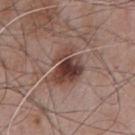Imaged during a routine full-body skin examination; the lesion was not biopsied and no histopathology is available.
On the chest.
Automated tile analysis of the lesion measured a mean CIELAB color near L≈41 a*≈20 b*≈23 and roughly 14 lightness units darker than nearby skin. It also reported an automated nevus-likeness rating near 95 out of 100 and lesion-presence confidence of about 100/100.
A male subject, aged 63 to 67.
Imaged with white-light lighting.
A 15 mm crop from a total-body photograph taken for skin-cancer surveillance.
Measured at roughly 4.5 mm in maximum diameter.